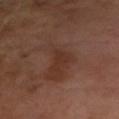  lesion_size:
    long_diameter_mm_approx: 3.5
  patient:
    sex: male
    age_approx: 65
  image:
    source: total-body photography crop
    field_of_view_mm: 15